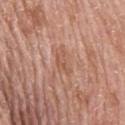Findings:
- follow-up · imaged on a skin check; not biopsied